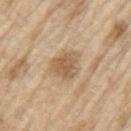Impression:
Recorded during total-body skin imaging; not selected for excision or biopsy.
Acquisition and patient details:
The tile uses white-light illumination. A male subject, aged approximately 70. The recorded lesion diameter is about 4 mm. A 15 mm crop from a total-body photograph taken for skin-cancer surveillance. The lesion is on the left upper arm. An algorithmic analysis of the crop reported an area of roughly 9.5 mm², an eccentricity of roughly 0.6, and a symmetry-axis asymmetry near 0.3. It also reported a nevus-likeness score of about 5/100 and a lesion-detection confidence of about 100/100.